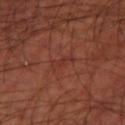Impression:
Recorded during total-body skin imaging; not selected for excision or biopsy.
Image and clinical context:
Captured under cross-polarized illumination. Approximately 3 mm at its widest. A male patient, approximately 70 years of age. On the left lower leg. Cropped from a total-body skin-imaging series; the visible field is about 15 mm. Automated image analysis of the tile measured a lesion area of about 3.5 mm², an outline eccentricity of about 0.95 (0 = round, 1 = elongated), and a symmetry-axis asymmetry near 0.25. The analysis additionally found a border-irregularity index near 3.5/10, a within-lesion color-variation index near 0/10, and a peripheral color-asymmetry measure near 0. The software also gave a nevus-likeness score of about 0/100 and a lesion-detection confidence of about 90/100.